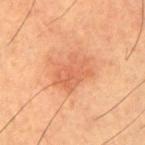No biopsy was performed on this lesion — it was imaged during a full skin examination and was not determined to be concerning.
A male patient approximately 65 years of age.
Measured at roughly 4.5 mm in maximum diameter.
Captured under cross-polarized illumination.
On the right upper arm.
A 15 mm close-up tile from a total-body photography series done for melanoma screening.